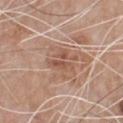Notes:
• notes: no biopsy performed (imaged during a skin exam)
• illumination: white-light
• image: 15 mm crop, total-body photography
• patient: male, roughly 80 years of age
• automated metrics: an area of roughly 4.5 mm², an eccentricity of roughly 0.65, and a symmetry-axis asymmetry near 0.6; about 8 CIELAB-L* units darker than the surrounding skin and a lesion-to-skin contrast of about 6 (normalized; higher = more distinct); a border-irregularity index near 8/10, internal color variation of about 0.5 on a 0–10 scale, and peripheral color asymmetry of about 0
• size: ≈3.5 mm
• anatomic site: the chest